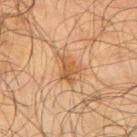workup — no biopsy performed (imaged during a skin exam)
anatomic site — the right upper arm
size — ≈4 mm
subject — male, approximately 65 years of age
image — total-body-photography crop, ~15 mm field of view
illumination — cross-polarized
image-analysis metrics — a nevus-likeness score of about 20/100 and a detector confidence of about 100 out of 100 that the crop contains a lesion